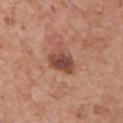Impression: Imaged during a routine full-body skin examination; the lesion was not biopsied and no histopathology is available. Context: A male subject, in their mid- to late 50s. The lesion-visualizer software estimated a mean CIELAB color near L≈46 a*≈24 b*≈27, roughly 13 lightness units darker than nearby skin, and a normalized border contrast of about 9.5. A roughly 15 mm field-of-view crop from a total-body skin photograph. Captured under white-light illumination. From the arm.